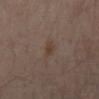A patient aged 53 to 57.
A 15 mm close-up tile from a total-body photography series done for melanoma screening.
The lesion is on the mid back.